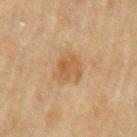Impression:
Part of a total-body skin-imaging series; this lesion was reviewed on a skin check and was not flagged for biopsy.
Clinical summary:
Cropped from a whole-body photographic skin survey; the tile spans about 15 mm. From the left upper arm. A male subject, aged approximately 70. Imaged with cross-polarized lighting. Longest diameter approximately 4 mm.